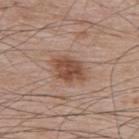Impression:
No biopsy was performed on this lesion — it was imaged during a full skin examination and was not determined to be concerning.
Image and clinical context:
Imaged with white-light lighting. The lesion-visualizer software estimated border irregularity of about 2.5 on a 0–10 scale, a color-variation rating of about 3.5/10, and peripheral color asymmetry of about 1. And it measured a classifier nevus-likeness of about 90/100 and lesion-presence confidence of about 100/100. The subject is a male aged around 65. The lesion is on the back. A roughly 15 mm field-of-view crop from a total-body skin photograph.